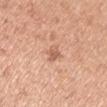biopsy status: catalogued during a skin exam; not biopsied
illumination: white-light illumination
subject: male, aged 48 to 52
size: ≈2.5 mm
image: 15 mm crop, total-body photography
anatomic site: the right upper arm